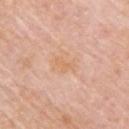tile lighting=white-light illumination | site=the upper back | imaging modality=15 mm crop, total-body photography | subject=male, roughly 75 years of age.